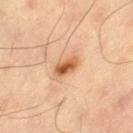- workup — no biopsy performed (imaged during a skin exam)
- lighting — cross-polarized
- patient — male, approximately 60 years of age
- location — the leg
- automated lesion analysis — roughly 14 lightness units darker than nearby skin and a lesion-to-skin contrast of about 9.5 (normalized; higher = more distinct); a border-irregularity rating of about 2/10 and internal color variation of about 7.5 on a 0–10 scale
- diameter — ≈3 mm
- acquisition — ~15 mm crop, total-body skin-cancer survey